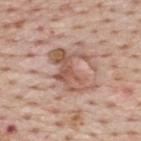acquisition: 15 mm crop, total-body photography
patient: male, about 75 years old
anatomic site: the upper back
illumination: white-light
lesion size: ~6 mm (longest diameter)